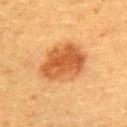body site=the upper back
patient=female, aged approximately 55
imaging modality=~15 mm tile from a whole-body skin photo